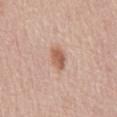Captured during whole-body skin photography for melanoma surveillance; the lesion was not biopsied. The lesion is on the mid back. This is a white-light tile. The total-body-photography lesion software estimated a border-irregularity rating of about 2/10, internal color variation of about 2 on a 0–10 scale, and peripheral color asymmetry of about 0.5. The software also gave a nevus-likeness score of about 85/100 and a detector confidence of about 100 out of 100 that the crop contains a lesion. A 15 mm close-up tile from a total-body photography series done for melanoma screening. A male subject in their 60s.